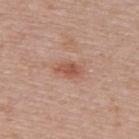• notes: total-body-photography surveillance lesion; no biopsy
• anatomic site: the back
• subject: female, roughly 60 years of age
• image source: 15 mm crop, total-body photography